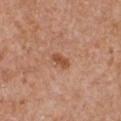This lesion was catalogued during total-body skin photography and was not selected for biopsy. Captured under white-light illumination. Located on the chest. A male patient in their mid-60s. A region of skin cropped from a whole-body photographic capture, roughly 15 mm wide. The recorded lesion diameter is about 2.5 mm. Automated tile analysis of the lesion measured a border-irregularity index near 3/10, a color-variation rating of about 0.5/10, and a peripheral color-asymmetry measure near 0.